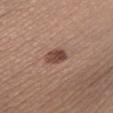biopsy status=total-body-photography surveillance lesion; no biopsy | tile lighting=white-light | location=the left lower leg | size=~3 mm (longest diameter) | imaging modality=~15 mm crop, total-body skin-cancer survey | patient=male, aged 33 to 37.